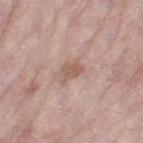The lesion was photographed on a routine skin check and not biopsied; there is no pathology result. A region of skin cropped from a whole-body photographic capture, roughly 15 mm wide. This is a white-light tile. Measured at roughly 3 mm in maximum diameter. The lesion is located on the left thigh. A female subject approximately 70 years of age. The lesion-visualizer software estimated an area of roughly 4 mm² and two-axis asymmetry of about 0.35. It also reported a border-irregularity rating of about 3.5/10 and internal color variation of about 1.5 on a 0–10 scale. The software also gave an automated nevus-likeness rating near 0 out of 100 and lesion-presence confidence of about 100/100.